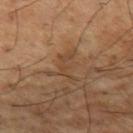{
  "biopsy_status": "not biopsied; imaged during a skin examination",
  "lesion_size": {
    "long_diameter_mm_approx": 3.5
  },
  "automated_metrics": {
    "cielab_L": 44,
    "cielab_a": 18,
    "cielab_b": 32,
    "vs_skin_darker_L": 6.0,
    "vs_skin_contrast_norm": 5.0,
    "border_irregularity_0_10": 9.0,
    "color_variation_0_10": 0.0,
    "peripheral_color_asymmetry": 0.0
  },
  "image": {
    "source": "total-body photography crop",
    "field_of_view_mm": 15
  },
  "patient": {
    "sex": "male",
    "age_approx": 65
  },
  "lighting": "cross-polarized",
  "site": "leg"
}